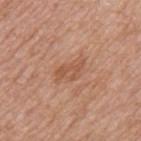<lesion>
  <biopsy_status>not biopsied; imaged during a skin examination</biopsy_status>
  <automated_metrics>
    <vs_skin_darker_L>7.0</vs_skin_darker_L>
    <vs_skin_contrast_norm>5.5</vs_skin_contrast_norm>
    <peripheral_color_asymmetry>0.5</peripheral_color_asymmetry>
    <nevus_likeness_0_100>5</nevus_likeness_0_100>
    <lesion_detection_confidence_0_100>100</lesion_detection_confidence_0_100>
  </automated_metrics>
  <lesion_size>
    <long_diameter_mm_approx>4.0</long_diameter_mm_approx>
  </lesion_size>
  <site>mid back</site>
  <lighting>white-light</lighting>
  <image>
    <source>total-body photography crop</source>
    <field_of_view_mm>15</field_of_view_mm>
  </image>
  <patient>
    <sex>male</sex>
    <age_approx>60</age_approx>
  </patient>
</lesion>